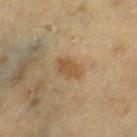workup = imaged on a skin check; not biopsied
location = the leg
patient = female, aged approximately 55
image source = ~15 mm crop, total-body skin-cancer survey